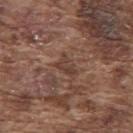  lighting: white-light
  site: upper back
  patient:
    sex: male
    age_approx: 75
  image:
    source: total-body photography crop
    field_of_view_mm: 15
  lesion_size:
    long_diameter_mm_approx: 2.5